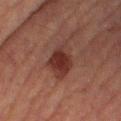workup: imaged on a skin check; not biopsied
subject: female, aged 53–57
site: the right thigh
image: ~15 mm tile from a whole-body skin photo
illumination: cross-polarized